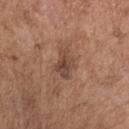Impression:
Part of a total-body skin-imaging series; this lesion was reviewed on a skin check and was not flagged for biopsy.
Clinical summary:
Imaged with white-light lighting. Automated tile analysis of the lesion measured a shape eccentricity near 0.75 and a shape-asymmetry score of about 0.35 (0 = symmetric). It also reported border irregularity of about 3.5 on a 0–10 scale, a color-variation rating of about 3.5/10, and peripheral color asymmetry of about 1. The analysis additionally found a classifier nevus-likeness of about 10/100. A 15 mm crop from a total-body photograph taken for skin-cancer surveillance. A male patient, roughly 50 years of age. Approximately 3.5 mm at its widest. The lesion is located on the head or neck.